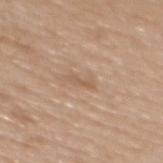Q: Was a biopsy performed?
A: catalogued during a skin exam; not biopsied